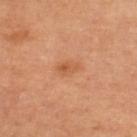notes: no biopsy performed (imaged during a skin exam) | patient: female, aged 58–62 | tile lighting: cross-polarized illumination | acquisition: total-body-photography crop, ~15 mm field of view | location: the right lower leg | automated metrics: a lesion area of about 4 mm², an outline eccentricity of about 0.85 (0 = round, 1 = elongated), and a symmetry-axis asymmetry near 0.2; a lesion color around L≈58 a*≈26 b*≈39 in CIELAB and a normalized border contrast of about 5.5.